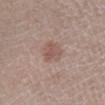biopsy status = imaged on a skin check; not biopsied | location = the left lower leg | lighting = white-light | subject = male, aged 58 to 62 | lesion diameter = about 3 mm | imaging modality = ~15 mm tile from a whole-body skin photo.